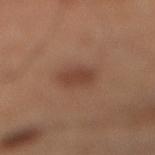Recorded during total-body skin imaging; not selected for excision or biopsy.
A male patient aged around 60.
Measured at roughly 3 mm in maximum diameter.
The lesion is located on the right lower leg.
A lesion tile, about 15 mm wide, cut from a 3D total-body photograph.
The lesion-visualizer software estimated an area of roughly 5 mm², an eccentricity of roughly 0.55, and a symmetry-axis asymmetry near 0.2. And it measured an average lesion color of about L≈40 a*≈21 b*≈27 (CIELAB). The analysis additionally found a border-irregularity index near 2/10 and a peripheral color-asymmetry measure near 0.5.